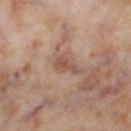Notes:
• biopsy status — total-body-photography surveillance lesion; no biopsy
• lesion diameter — ≈3 mm
• subject — female, about 55 years old
• automated lesion analysis — a lesion area of about 3.5 mm², an outline eccentricity of about 0.9 (0 = round, 1 = elongated), and a shape-asymmetry score of about 0.45 (0 = symmetric); a mean CIELAB color near L≈52 a*≈20 b*≈28, about 9 CIELAB-L* units darker than the surrounding skin, and a lesion-to-skin contrast of about 7 (normalized; higher = more distinct); border irregularity of about 5 on a 0–10 scale and a color-variation rating of about 1.5/10
• location — the right lower leg
• image source — 15 mm crop, total-body photography